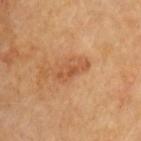Case summary:
* workup: no biopsy performed (imaged during a skin exam)
* image source: 15 mm crop, total-body photography
* site: the back
* tile lighting: cross-polarized illumination
* size: ~4 mm (longest diameter)
* subject: male, in their 60s